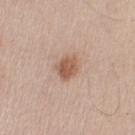The lesion was tiled from a total-body skin photograph and was not biopsied. The subject is a male aged around 50. Cropped from a whole-body photographic skin survey; the tile spans about 15 mm. The total-body-photography lesion software estimated a lesion area of about 5.5 mm², a shape eccentricity near 0.65, and a symmetry-axis asymmetry near 0.2. The recorded lesion diameter is about 3 mm. The lesion is located on the left upper arm.